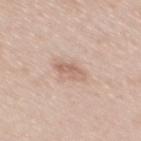The lesion was tiled from a total-body skin photograph and was not biopsied. The tile uses white-light illumination. A male subject, aged 33 to 37. The lesion is located on the mid back. Cropped from a whole-body photographic skin survey; the tile spans about 15 mm.